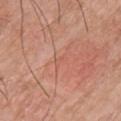Q: Was a biopsy performed?
A: no biopsy performed (imaged during a skin exam)
Q: What are the patient's age and sex?
A: female, approximately 60 years of age
Q: Lesion size?
A: ≈2 mm
Q: What lighting was used for the tile?
A: white-light illumination
Q: How was this image acquired?
A: ~15 mm tile from a whole-body skin photo
Q: Automated lesion metrics?
A: a footprint of about 1.5 mm² and a shape-asymmetry score of about 0.4 (0 = symmetric); about 4 CIELAB-L* units darker than the surrounding skin and a normalized border contrast of about 3; border irregularity of about 4 on a 0–10 scale and peripheral color asymmetry of about 0
Q: Where on the body is the lesion?
A: the chest